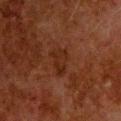No biopsy was performed on this lesion — it was imaged during a full skin examination and was not determined to be concerning.
Automated tile analysis of the lesion measured an area of roughly 5.5 mm², an outline eccentricity of about 0.85 (0 = round, 1 = elongated), and two-axis asymmetry of about 0.4. The software also gave a nevus-likeness score of about 0/100.
Imaged with cross-polarized lighting.
Located on the head or neck.
This image is a 15 mm lesion crop taken from a total-body photograph.
About 3.5 mm across.
A male patient in their 80s.